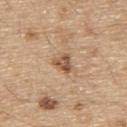notes — no biopsy performed (imaged during a skin exam) | body site — the upper back | patient — male, aged 68 to 72 | illumination — white-light | image source — ~15 mm crop, total-body skin-cancer survey | size — ~2.5 mm (longest diameter).